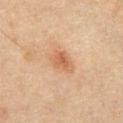  biopsy_status: not biopsied; imaged during a skin examination
  lighting: cross-polarized
  lesion_size:
    long_diameter_mm_approx: 3.5
  patient:
    sex: male
    age_approx: 65
  image:
    source: total-body photography crop
    field_of_view_mm: 15
  site: abdomen